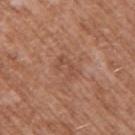Impression:
No biopsy was performed on this lesion — it was imaged during a full skin examination and was not determined to be concerning.
Background:
A male subject in their 60s. An algorithmic analysis of the crop reported a symmetry-axis asymmetry near 0.5. And it measured a border-irregularity index near 7/10 and a within-lesion color-variation index near 0/10. And it measured a nevus-likeness score of about 0/100 and a lesion-detection confidence of about 100/100. Approximately 3 mm at its widest. A 15 mm close-up tile from a total-body photography series done for melanoma screening. On the left upper arm. The tile uses white-light illumination.